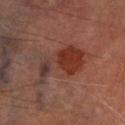Q: Was a biopsy performed?
A: total-body-photography surveillance lesion; no biopsy
Q: Patient demographics?
A: male, aged 68–72
Q: How large is the lesion?
A: about 7 mm
Q: Lesion location?
A: the right lower leg
Q: What is the imaging modality?
A: 15 mm crop, total-body photography
Q: Illumination type?
A: cross-polarized
Q: Automated lesion metrics?
A: an average lesion color of about L≈28 a*≈20 b*≈22 (CIELAB), about 7 CIELAB-L* units darker than the surrounding skin, and a normalized border contrast of about 7.5; a nevus-likeness score of about 100/100 and lesion-presence confidence of about 100/100